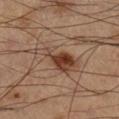Q: Is there a histopathology result?
A: no biopsy performed (imaged during a skin exam)
Q: Illumination type?
A: cross-polarized
Q: How was this image acquired?
A: ~15 mm crop, total-body skin-cancer survey
Q: What are the patient's age and sex?
A: male, aged 58–62
Q: Where on the body is the lesion?
A: the right lower leg
Q: Automated lesion metrics?
A: a lesion–skin lightness drop of about 11; a border-irregularity rating of about 6.5/10, internal color variation of about 6.5 on a 0–10 scale, and a peripheral color-asymmetry measure near 2; a lesion-detection confidence of about 100/100